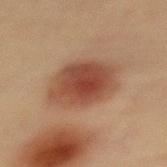• biopsy status: total-body-photography surveillance lesion; no biopsy
• imaging modality: ~15 mm crop, total-body skin-cancer survey
• patient: female, in their mid-60s
• tile lighting: cross-polarized illumination
• location: the back
• automated metrics: an area of roughly 22 mm², a shape eccentricity near 0.6, and a shape-asymmetry score of about 0.1 (0 = symmetric); a mean CIELAB color near L≈40 a*≈21 b*≈27, about 11 CIELAB-L* units darker than the surrounding skin, and a normalized border contrast of about 9